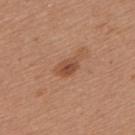This lesion was catalogued during total-body skin photography and was not selected for biopsy. Automated image analysis of the tile measured a mean CIELAB color near L≈49 a*≈23 b*≈32, about 10 CIELAB-L* units darker than the surrounding skin, and a normalized lesion–skin contrast near 7. The analysis additionally found a border-irregularity rating of about 2.5/10. This is a white-light tile. Cropped from a whole-body photographic skin survey; the tile spans about 15 mm. From the back. The patient is a female about 45 years old. Measured at roughly 2.5 mm in maximum diameter.